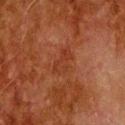{"biopsy_status": "not biopsied; imaged during a skin examination", "image": {"source": "total-body photography crop", "field_of_view_mm": 15}, "automated_metrics": {"area_mm2_approx": 5.5, "eccentricity": 0.85, "cielab_L": 29, "cielab_a": 24, "cielab_b": 29, "vs_skin_darker_L": 5.0, "vs_skin_contrast_norm": 5.0, "border_irregularity_0_10": 3.5, "color_variation_0_10": 2.0, "peripheral_color_asymmetry": 0.5, "nevus_likeness_0_100": 0, "lesion_detection_confidence_0_100": 100}, "lighting": "cross-polarized", "site": "head or neck", "lesion_size": {"long_diameter_mm_approx": 3.5}, "patient": {"sex": "male", "age_approx": 80}}